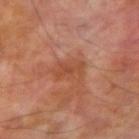Recorded during total-body skin imaging; not selected for excision or biopsy. A region of skin cropped from a whole-body photographic capture, roughly 15 mm wide. On the right upper arm. The patient is a male aged approximately 70. Approximately 3.5 mm at its widest. Captured under cross-polarized illumination.